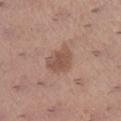Part of a total-body skin-imaging series; this lesion was reviewed on a skin check and was not flagged for biopsy.
From the right lower leg.
The tile uses white-light illumination.
Cropped from a total-body skin-imaging series; the visible field is about 15 mm.
About 3.5 mm across.
A female patient, aged around 65.